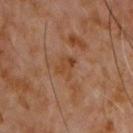No biopsy was performed on this lesion — it was imaged during a full skin examination and was not determined to be concerning. The total-body-photography lesion software estimated a mean CIELAB color near L≈41 a*≈20 b*≈32. The analysis additionally found a border-irregularity index near 3.5/10, a color-variation rating of about 2.5/10, and peripheral color asymmetry of about 1. Longest diameter approximately 2.5 mm. Cropped from a whole-body photographic skin survey; the tile spans about 15 mm. A male patient, aged 58 to 62. The lesion is located on the chest.